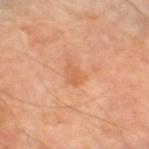Impression:
No biopsy was performed on this lesion — it was imaged during a full skin examination and was not determined to be concerning.
Acquisition and patient details:
The patient is a male aged approximately 70. The lesion is on the left thigh. Longest diameter approximately 3 mm. Cropped from a total-body skin-imaging series; the visible field is about 15 mm.